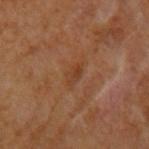Notes:
• workup · no biopsy performed (imaged during a skin exam)